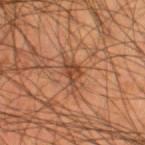The lesion was photographed on a routine skin check and not biopsied; there is no pathology result. Located on the right thigh. The subject is a male approximately 55 years of age. Cropped from a total-body skin-imaging series; the visible field is about 15 mm. Longest diameter approximately 4 mm.